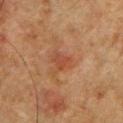This lesion was catalogued during total-body skin photography and was not selected for biopsy. The tile uses cross-polarized illumination. The lesion is located on the chest. A male patient, aged 58 to 62. An algorithmic analysis of the crop reported a lesion area of about 4.5 mm², an eccentricity of roughly 0.7, and a shape-asymmetry score of about 0.35 (0 = symmetric). The software also gave a mean CIELAB color near L≈39 a*≈22 b*≈29 and a normalized lesion–skin contrast near 5.5. The analysis additionally found border irregularity of about 3.5 on a 0–10 scale, internal color variation of about 3 on a 0–10 scale, and radial color variation of about 1. And it measured an automated nevus-likeness rating near 15 out of 100. A lesion tile, about 15 mm wide, cut from a 3D total-body photograph.